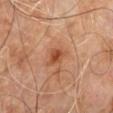Case summary:
• lighting — cross-polarized
• body site — the lower back
• subject — male, about 60 years old
• image — 15 mm crop, total-body photography